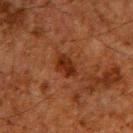The total-body-photography lesion software estimated an area of roughly 4.5 mm² and two-axis asymmetry of about 0.25. It also reported a mean CIELAB color near L≈22 a*≈22 b*≈27 and a normalized border contrast of about 9. The software also gave a border-irregularity index near 2.5/10 and a color-variation rating of about 2.5/10. The software also gave an automated nevus-likeness rating near 90 out of 100 and a detector confidence of about 100 out of 100 that the crop contains a lesion.
A male subject, in their 60s.
Measured at roughly 3 mm in maximum diameter.
The lesion is located on the upper back.
A close-up tile cropped from a whole-body skin photograph, about 15 mm across.